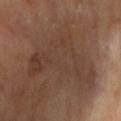This lesion was catalogued during total-body skin photography and was not selected for biopsy. From the right upper arm. About 9 mm across. A female patient, aged around 65. Captured under cross-polarized illumination. A roughly 15 mm field-of-view crop from a total-body skin photograph.